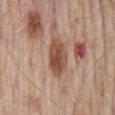Q: Was a biopsy performed?
A: no biopsy performed (imaged during a skin exam)
Q: What are the patient's age and sex?
A: male, aged 53 to 57
Q: Lesion location?
A: the mid back
Q: What is the lesion's diameter?
A: ~4.5 mm (longest diameter)
Q: What lighting was used for the tile?
A: white-light illumination
Q: What is the imaging modality?
A: ~15 mm tile from a whole-body skin photo
Q: Automated lesion metrics?
A: an area of roughly 10 mm² and a symmetry-axis asymmetry near 0.15; a mean CIELAB color near L≈50 a*≈21 b*≈29 and a normalized lesion–skin contrast near 9; a classifier nevus-likeness of about 0/100 and a lesion-detection confidence of about 95/100